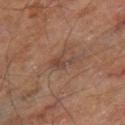| key | value |
|---|---|
| follow-up | total-body-photography surveillance lesion; no biopsy |
| lesion size | ≈3.5 mm |
| patient | male, approximately 70 years of age |
| imaging modality | ~15 mm crop, total-body skin-cancer survey |
| tile lighting | cross-polarized |
| anatomic site | the right thigh |